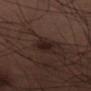The lesion was photographed on a routine skin check and not biopsied; there is no pathology result. The lesion's longest dimension is about 3 mm. The lesion is on the left thigh. A 15 mm crop from a total-body photograph taken for skin-cancer surveillance. The lesion-visualizer software estimated a border-irregularity index near 3.5/10 and a peripheral color-asymmetry measure near 1.5. This is a cross-polarized tile. The subject is a male roughly 40 years of age.